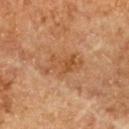No biopsy was performed on this lesion — it was imaged during a full skin examination and was not determined to be concerning. About 5 mm across. A 15 mm close-up tile from a total-body photography series done for melanoma screening. An algorithmic analysis of the crop reported a lesion color around L≈48 a*≈22 b*≈36 in CIELAB and a lesion-to-skin contrast of about 6.5 (normalized; higher = more distinct). And it measured a border-irregularity index near 6.5/10, internal color variation of about 4 on a 0–10 scale, and radial color variation of about 1.5. Located on the upper back. The tile uses cross-polarized illumination. The subject is a female approximately 60 years of age.